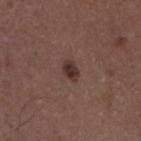From the right upper arm. The tile uses white-light illumination. The patient is a male in their 50s. A lesion tile, about 15 mm wide, cut from a 3D total-body photograph. Longest diameter approximately 2.5 mm.